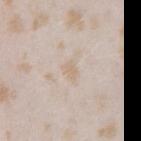Assessment: The lesion was tiled from a total-body skin photograph and was not biopsied. Clinical summary: The lesion is on the right upper arm. Approximately 2.5 mm at its widest. A close-up tile cropped from a whole-body skin photograph, about 15 mm across. An algorithmic analysis of the crop reported a footprint of about 3 mm² and an outline eccentricity of about 0.8 (0 = round, 1 = elongated). The software also gave a mean CIELAB color near L≈69 a*≈11 b*≈27. And it measured a border-irregularity rating of about 3.5/10, internal color variation of about 1 on a 0–10 scale, and radial color variation of about 0.5. And it measured a classifier nevus-likeness of about 0/100 and a detector confidence of about 90 out of 100 that the crop contains a lesion. The patient is a female roughly 25 years of age.